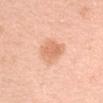<tbp_lesion>
<biopsy_status>not biopsied; imaged during a skin examination</biopsy_status>
<site>left upper arm</site>
<image>
  <source>total-body photography crop</source>
  <field_of_view_mm>15</field_of_view_mm>
</image>
<lesion_size>
  <long_diameter_mm_approx>4.0</long_diameter_mm_approx>
</lesion_size>
<automated_metrics>
  <area_mm2_approx>8.0</area_mm2_approx>
  <shape_asymmetry>0.3</shape_asymmetry>
  <border_irregularity_0_10>2.5</border_irregularity_0_10>
  <peripheral_color_asymmetry>1.0</peripheral_color_asymmetry>
</automated_metrics>
<patient>
  <sex>female</sex>
  <age_approx>40</age_approx>
</patient>
</tbp_lesion>